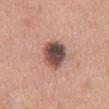Longest diameter approximately 4.5 mm. This is a white-light tile. An algorithmic analysis of the crop reported a lesion color around L≈48 a*≈20 b*≈23 in CIELAB and a normalized lesion–skin contrast near 12.5. It also reported internal color variation of about 9 on a 0–10 scale and peripheral color asymmetry of about 2.5. The lesion is located on the mid back. This image is a 15 mm lesion crop taken from a total-body photograph. The patient is a female aged 38 to 42.